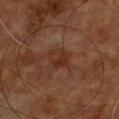Impression:
The lesion was photographed on a routine skin check and not biopsied; there is no pathology result.
Acquisition and patient details:
A 15 mm close-up extracted from a 3D total-body photography capture. The lesion is on the chest. A male subject approximately 60 years of age.